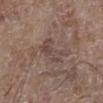workup=total-body-photography surveillance lesion; no biopsy
anatomic site=the left lower leg
image=~15 mm crop, total-body skin-cancer survey
size=~4.5 mm (longest diameter)
patient=male, roughly 65 years of age